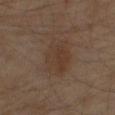Recorded during total-body skin imaging; not selected for excision or biopsy. A 15 mm close-up tile from a total-body photography series done for melanoma screening. From the right thigh. A male subject in their 60s.